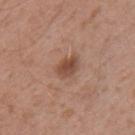The lesion was tiled from a total-body skin photograph and was not biopsied. A 15 mm close-up extracted from a 3D total-body photography capture. Automated tile analysis of the lesion measured an area of roughly 6 mm², an eccentricity of roughly 0.75, and a shape-asymmetry score of about 0.2 (0 = symmetric). It also reported a nevus-likeness score of about 75/100 and a lesion-detection confidence of about 100/100. The subject is a male aged approximately 45. The lesion is located on the mid back. About 3.5 mm across. The tile uses white-light illumination.